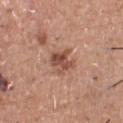follow-up: no biopsy performed (imaged during a skin exam) | lesion diameter: about 3 mm | automated metrics: a lesion area of about 6.5 mm², an outline eccentricity of about 0.5 (0 = round, 1 = elongated), and a shape-asymmetry score of about 0.35 (0 = symmetric) | illumination: white-light illumination | image source: ~15 mm crop, total-body skin-cancer survey | patient: male, in their mid- to late 40s | body site: the chest.